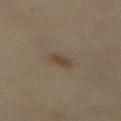notes: catalogued during a skin exam; not biopsied
location: the front of the torso
subject: female, roughly 60 years of age
automated metrics: border irregularity of about 2 on a 0–10 scale, a color-variation rating of about 2.5/10, and a peripheral color-asymmetry measure near 1
imaging modality: ~15 mm crop, total-body skin-cancer survey
illumination: cross-polarized illumination